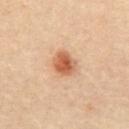Part of a total-body skin-imaging series; this lesion was reviewed on a skin check and was not flagged for biopsy. On the abdomen. A female subject in their mid- to late 60s. Longest diameter approximately 3 mm. Imaged with cross-polarized lighting. A roughly 15 mm field-of-view crop from a total-body skin photograph. The lesion-visualizer software estimated a shape eccentricity near 0.55. The analysis additionally found a mean CIELAB color near L≈58 a*≈25 b*≈36, a lesion–skin lightness drop of about 14, and a lesion-to-skin contrast of about 9 (normalized; higher = more distinct). And it measured a nevus-likeness score of about 100/100 and a lesion-detection confidence of about 100/100.